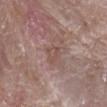{
  "biopsy_status": "not biopsied; imaged during a skin examination",
  "site": "right forearm",
  "patient": {
    "sex": "male",
    "age_approx": 75
  },
  "lighting": "white-light",
  "automated_metrics": {
    "area_mm2_approx": 3.0,
    "shape_asymmetry": 0.3,
    "cielab_L": 50,
    "cielab_a": 18,
    "cielab_b": 23,
    "vs_skin_darker_L": 5.0,
    "vs_skin_contrast_norm": 4.5,
    "border_irregularity_0_10": 3.0,
    "color_variation_0_10": 2.0,
    "peripheral_color_asymmetry": 0.5,
    "nevus_likeness_0_100": 0,
    "lesion_detection_confidence_0_100": 95
  },
  "image": {
    "source": "total-body photography crop",
    "field_of_view_mm": 15
  },
  "lesion_size": {
    "long_diameter_mm_approx": 2.5
  }
}